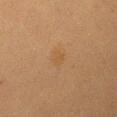{
  "biopsy_status": "not biopsied; imaged during a skin examination",
  "patient": {
    "sex": "female",
    "age_approx": 55
  },
  "image": {
    "source": "total-body photography crop",
    "field_of_view_mm": 15
  },
  "site": "back",
  "lighting": "cross-polarized"
}